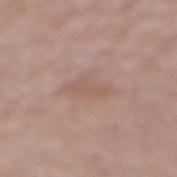This lesion was catalogued during total-body skin photography and was not selected for biopsy.
A lesion tile, about 15 mm wide, cut from a 3D total-body photograph.
Approximately 4.5 mm at its widest.
A male patient, in their mid- to late 80s or older.
The tile uses white-light illumination.
The lesion is located on the mid back.